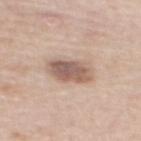notes=no biopsy performed (imaged during a skin exam)
location=the upper back
diameter=~4.5 mm (longest diameter)
subject=female, aged approximately 65
image=15 mm crop, total-body photography
tile lighting=white-light